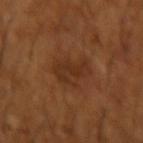No biopsy was performed on this lesion — it was imaged during a full skin examination and was not determined to be concerning.
This image is a 15 mm lesion crop taken from a total-body photograph.
A male patient, in their mid-60s.
The lesion is on the right forearm.
This is a cross-polarized tile.
Approximately 4.5 mm at its widest.